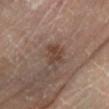No biopsy was performed on this lesion — it was imaged during a full skin examination and was not determined to be concerning. The lesion is on the left lower leg. A roughly 15 mm field-of-view crop from a total-body skin photograph. A male subject, approximately 70 years of age. The total-body-photography lesion software estimated a lesion area of about 5.5 mm², a shape eccentricity near 0.75, and two-axis asymmetry of about 0.3. And it measured a lesion color around L≈40 a*≈16 b*≈24 in CIELAB, a lesion–skin lightness drop of about 8, and a normalized lesion–skin contrast near 7. And it measured border irregularity of about 3 on a 0–10 scale, internal color variation of about 3 on a 0–10 scale, and a peripheral color-asymmetry measure near 1. The analysis additionally found a lesion-detection confidence of about 100/100. The tile uses cross-polarized illumination. About 3 mm across.